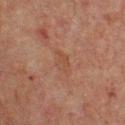Assessment:
This lesion was catalogued during total-body skin photography and was not selected for biopsy.
Image and clinical context:
A male patient aged around 65. Imaged with cross-polarized lighting. The lesion is on the upper back. This image is a 15 mm lesion crop taken from a total-body photograph. Measured at roughly 3 mm in maximum diameter.